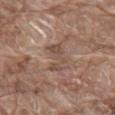Clinical impression:
Captured during whole-body skin photography for melanoma surveillance; the lesion was not biopsied.
Image and clinical context:
Imaged with white-light lighting. Cropped from a total-body skin-imaging series; the visible field is about 15 mm. The subject is a male aged 78 to 82. The recorded lesion diameter is about 4 mm. On the mid back.